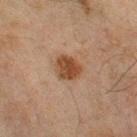{
  "biopsy_status": "not biopsied; imaged during a skin examination",
  "lesion_size": {
    "long_diameter_mm_approx": 3.0
  },
  "image": {
    "source": "total-body photography crop",
    "field_of_view_mm": 15
  },
  "lighting": "cross-polarized",
  "site": "right lower leg",
  "patient": {
    "sex": "male",
    "age_approx": 45
  }
}